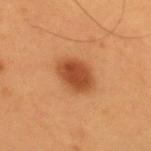workup — imaged on a skin check; not biopsied
image-analysis metrics — an area of roughly 10 mm² and a shape-asymmetry score of about 0.15 (0 = symmetric); a lesion color around L≈47 a*≈28 b*≈39 in CIELAB, roughly 13 lightness units darker than nearby skin, and a lesion-to-skin contrast of about 9.5 (normalized; higher = more distinct); border irregularity of about 1.5 on a 0–10 scale, a color-variation rating of about 3/10, and peripheral color asymmetry of about 1
subject — male, aged 53 to 57
image source — total-body-photography crop, ~15 mm field of view
size — about 4.5 mm
illumination — cross-polarized
body site — the upper back